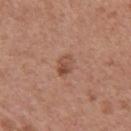{"biopsy_status": "not biopsied; imaged during a skin examination", "patient": {"sex": "male", "age_approx": 45}, "lighting": "white-light", "lesion_size": {"long_diameter_mm_approx": 3.0}, "image": {"source": "total-body photography crop", "field_of_view_mm": 15}, "automated_metrics": {"shape_asymmetry": 0.3, "border_irregularity_0_10": 2.5, "color_variation_0_10": 6.0, "peripheral_color_asymmetry": 2.5, "nevus_likeness_0_100": 50, "lesion_detection_confidence_0_100": 100}, "site": "left upper arm"}